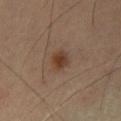| key | value |
|---|---|
| workup | no biopsy performed (imaged during a skin exam) |
| location | the left thigh |
| automated metrics | a classifier nevus-likeness of about 95/100 and a lesion-detection confidence of about 100/100 |
| imaging modality | ~15 mm crop, total-body skin-cancer survey |
| size | about 3 mm |
| patient | male, in their mid-50s |
| illumination | cross-polarized |